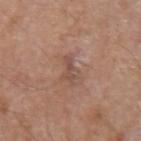Assessment: Imaged during a routine full-body skin examination; the lesion was not biopsied and no histopathology is available. Acquisition and patient details: The total-body-photography lesion software estimated a lesion color around L≈50 a*≈20 b*≈26 in CIELAB and a normalized border contrast of about 5.5. And it measured a border-irregularity rating of about 6/10. The software also gave an automated nevus-likeness rating near 0 out of 100 and a detector confidence of about 100 out of 100 that the crop contains a lesion. The recorded lesion diameter is about 3 mm. On the arm. Captured under white-light illumination. A region of skin cropped from a whole-body photographic capture, roughly 15 mm wide. A male subject aged around 80.